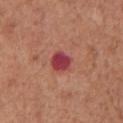Q: Was this lesion biopsied?
A: no biopsy performed (imaged during a skin exam)
Q: Automated lesion metrics?
A: a mean CIELAB color near L≈42 a*≈37 b*≈22, about 14 CIELAB-L* units darker than the surrounding skin, and a lesion-to-skin contrast of about 11.5 (normalized; higher = more distinct); a nevus-likeness score of about 0/100 and a detector confidence of about 100 out of 100 that the crop contains a lesion
Q: Lesion location?
A: the chest
Q: How was the tile lit?
A: white-light illumination
Q: How was this image acquired?
A: ~15 mm tile from a whole-body skin photo
Q: Lesion size?
A: ≈2.5 mm
Q: Patient demographics?
A: male, in their mid- to late 60s